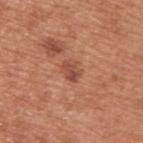Q: Was a biopsy performed?
A: total-body-photography surveillance lesion; no biopsy
Q: Who is the patient?
A: male, aged 63 to 67
Q: Lesion location?
A: the back
Q: Lesion size?
A: ~2.5 mm (longest diameter)
Q: How was this image acquired?
A: ~15 mm crop, total-body skin-cancer survey
Q: Illumination type?
A: white-light